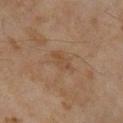biopsy status: total-body-photography surveillance lesion; no biopsy | tile lighting: cross-polarized illumination | acquisition: 15 mm crop, total-body photography | subject: female, in their 60s | diameter: ~4 mm (longest diameter) | body site: the right lower leg.